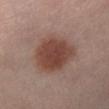Clinical impression:
Captured during whole-body skin photography for melanoma surveillance; the lesion was not biopsied.
Clinical summary:
A close-up tile cropped from a whole-body skin photograph, about 15 mm across. The total-body-photography lesion software estimated a lesion–skin lightness drop of about 11 and a normalized lesion–skin contrast near 9.5. The analysis additionally found a border-irregularity rating of about 2/10 and a within-lesion color-variation index near 3/10. A male subject aged approximately 40. The lesion is located on the leg. The tile uses cross-polarized illumination. About 5 mm across.